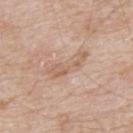biopsy status — imaged on a skin check; not biopsied
subject — male, roughly 65 years of age
location — the back
acquisition — ~15 mm tile from a whole-body skin photo
automated lesion analysis — a lesion area of about 7.5 mm² and a symmetry-axis asymmetry near 0.45
size — about 5.5 mm
illumination — white-light illumination